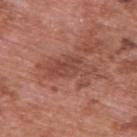Notes:
- follow-up — no biopsy performed (imaged during a skin exam)
- image source — 15 mm crop, total-body photography
- tile lighting — white-light illumination
- subject — male, aged around 70
- size — ≈6.5 mm
- image-analysis metrics — a mean CIELAB color near L≈47 a*≈25 b*≈28 and roughly 9 lightness units darker than nearby skin; a nevus-likeness score of about 0/100 and a lesion-detection confidence of about 100/100
- body site — the back